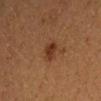Assessment:
Imaged during a routine full-body skin examination; the lesion was not biopsied and no histopathology is available.
Context:
Approximately 2.5 mm at its widest. Imaged with cross-polarized lighting. The total-body-photography lesion software estimated an eccentricity of roughly 0.35. The analysis additionally found an automated nevus-likeness rating near 85 out of 100 and lesion-presence confidence of about 100/100. A 15 mm crop from a total-body photograph taken for skin-cancer surveillance. From the left forearm. A female subject about 40 years old.